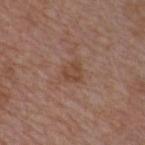Recorded during total-body skin imaging; not selected for excision or biopsy.
A male subject aged 48 to 52.
A 15 mm close-up extracted from a 3D total-body photography capture.
This is a white-light tile.
The total-body-photography lesion software estimated a shape eccentricity near 0.5 and a symmetry-axis asymmetry near 0.3. The analysis additionally found a border-irregularity rating of about 3/10, a color-variation rating of about 3/10, and radial color variation of about 1. The software also gave an automated nevus-likeness rating near 0 out of 100 and a detector confidence of about 100 out of 100 that the crop contains a lesion.
About 2.5 mm across.
The lesion is located on the chest.